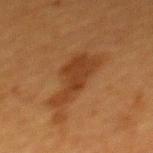Case summary:
– follow-up — no biopsy performed (imaged during a skin exam)
– image — total-body-photography crop, ~15 mm field of view
– location — the upper back
– subject — female, aged approximately 40
– lesion size — about 6 mm